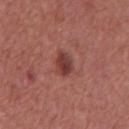Assessment:
This lesion was catalogued during total-body skin photography and was not selected for biopsy.
Acquisition and patient details:
Located on the mid back. Measured at roughly 2.5 mm in maximum diameter. The patient is a male about 65 years old. This is a white-light tile. Cropped from a total-body skin-imaging series; the visible field is about 15 mm.